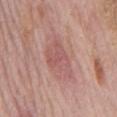{"biopsy_status": "not biopsied; imaged during a skin examination", "image": {"source": "total-body photography crop", "field_of_view_mm": 15}, "lesion_size": {"long_diameter_mm_approx": 4.5}, "lighting": "white-light", "patient": {"sex": "male", "age_approx": 75}, "site": "mid back"}